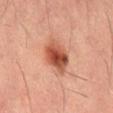Findings:
– follow-up · catalogued during a skin exam; not biopsied